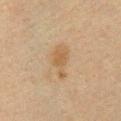Imaged during a routine full-body skin examination; the lesion was not biopsied and no histopathology is available.
From the front of the torso.
A female patient aged 38 to 42.
A region of skin cropped from a whole-body photographic capture, roughly 15 mm wide.
The tile uses cross-polarized illumination.
The recorded lesion diameter is about 4.5 mm.